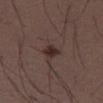biopsy status: imaged on a skin check; not biopsied | lighting: white-light illumination | subject: male, aged 48–52 | anatomic site: the leg | size: about 2.5 mm | imaging modality: total-body-photography crop, ~15 mm field of view.